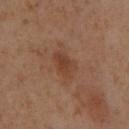The lesion was photographed on a routine skin check and not biopsied; there is no pathology result.
This is a cross-polarized tile.
A female patient, about 55 years old.
The lesion is located on the right lower leg.
This image is a 15 mm lesion crop taken from a total-body photograph.